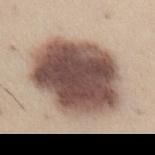follow-up: total-body-photography surveillance lesion; no biopsy
lesion diameter: ≈10 mm
lighting: white-light illumination
acquisition: 15 mm crop, total-body photography
anatomic site: the abdomen
patient: male, approximately 35 years of age